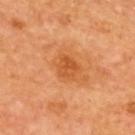<lesion>
  <biopsy_status>not biopsied; imaged during a skin examination</biopsy_status>
  <image>
    <source>total-body photography crop</source>
    <field_of_view_mm>15</field_of_view_mm>
  </image>
  <lesion_size>
    <long_diameter_mm_approx>2.5</long_diameter_mm_approx>
  </lesion_size>
  <patient>
    <sex>male</sex>
    <age_approx>65</age_approx>
  </patient>
  <site>upper back</site>
  <lighting>cross-polarized</lighting>
</lesion>